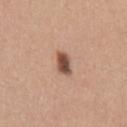Assessment: No biopsy was performed on this lesion — it was imaged during a full skin examination and was not determined to be concerning. Background: The tile uses white-light illumination. Measured at roughly 3 mm in maximum diameter. A female patient aged 23–27. A region of skin cropped from a whole-body photographic capture, roughly 15 mm wide. Located on the arm.